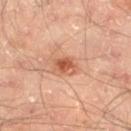Part of a total-body skin-imaging series; this lesion was reviewed on a skin check and was not flagged for biopsy.
Automated tile analysis of the lesion measured a mean CIELAB color near L≈56 a*≈27 b*≈34 and a lesion-to-skin contrast of about 7.5 (normalized; higher = more distinct).
A male patient, aged around 65.
The lesion's longest dimension is about 2.5 mm.
This is a cross-polarized tile.
This image is a 15 mm lesion crop taken from a total-body photograph.
The lesion is located on the right thigh.